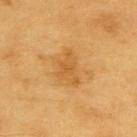An algorithmic analysis of the crop reported a color-variation rating of about 2.5/10 and radial color variation of about 0.5.
The lesion is located on the upper back.
A lesion tile, about 15 mm wide, cut from a 3D total-body photograph.
Measured at roughly 4 mm in maximum diameter.
A male patient, aged around 60.
Captured under cross-polarized illumination.